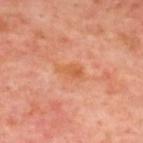Q: Was a biopsy performed?
A: total-body-photography surveillance lesion; no biopsy
Q: Where on the body is the lesion?
A: the upper back
Q: What is the imaging modality?
A: ~15 mm tile from a whole-body skin photo
Q: Patient demographics?
A: aged 53–57